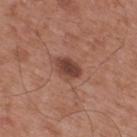<lesion>
<biopsy_status>not biopsied; imaged during a skin examination</biopsy_status>
<automated_metrics>
  <cielab_L>42</cielab_L>
  <cielab_a>22</cielab_a>
  <cielab_b>26</cielab_b>
  <vs_skin_darker_L>12.0</vs_skin_darker_L>
  <vs_skin_contrast_norm>9.5</vs_skin_contrast_norm>
  <border_irregularity_0_10>2.0</border_irregularity_0_10>
  <color_variation_0_10>2.5</color_variation_0_10>
  <nevus_likeness_0_100>70</nevus_likeness_0_100>
</automated_metrics>
<patient>
  <sex>male</sex>
  <age_approx>55</age_approx>
</patient>
<image>
  <source>total-body photography crop</source>
  <field_of_view_mm>15</field_of_view_mm>
</image>
<lesion_size>
  <long_diameter_mm_approx>3.5</long_diameter_mm_approx>
</lesion_size>
<lighting>white-light</lighting>
<site>upper back</site>
</lesion>